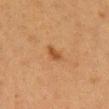{"biopsy_status": "not biopsied; imaged during a skin examination", "automated_metrics": {"area_mm2_approx": 3.0, "eccentricity": 0.85, "shape_asymmetry": 0.35, "border_irregularity_0_10": 3.0, "color_variation_0_10": 1.0, "peripheral_color_asymmetry": 0.5}, "patient": {"sex": "female", "age_approx": 40}, "site": "head or neck", "lesion_size": {"long_diameter_mm_approx": 2.5}, "image": {"source": "total-body photography crop", "field_of_view_mm": 15}, "lighting": "cross-polarized"}